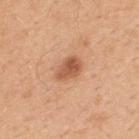Automated tile analysis of the lesion measured an outline eccentricity of about 0.75 (0 = round, 1 = elongated). It also reported a normalized lesion–skin contrast near 8. The analysis additionally found a nevus-likeness score of about 80/100 and lesion-presence confidence of about 100/100. Located on the upper back. A male subject aged 48 to 52. A close-up tile cropped from a whole-body skin photograph, about 15 mm across. The lesion's longest dimension is about 3.5 mm.